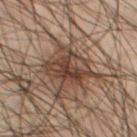Notes:
* workup · total-body-photography surveillance lesion; no biopsy
* image · 15 mm crop, total-body photography
* body site · the leg
* patient · male, approximately 50 years of age